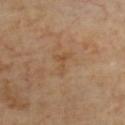• workup: total-body-photography surveillance lesion; no biopsy
• imaging modality: 15 mm crop, total-body photography
• size: ~3 mm (longest diameter)
• subject: female
• TBP lesion metrics: a footprint of about 3.5 mm² and a shape eccentricity near 0.8
• illumination: cross-polarized illumination
• site: the chest